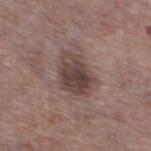follow-up: catalogued during a skin exam; not biopsied | automated metrics: a lesion area of about 13 mm² and a symmetry-axis asymmetry near 0.25; a lesion color around L≈42 a*≈16 b*≈19 in CIELAB, a lesion–skin lightness drop of about 12, and a normalized border contrast of about 9.5; a border-irregularity index near 2.5/10 and radial color variation of about 2; a classifier nevus-likeness of about 40/100 and lesion-presence confidence of about 100/100 | illumination: white-light | image: ~15 mm tile from a whole-body skin photo | location: the left thigh | subject: male, aged around 75 | lesion diameter: ≈5.5 mm.